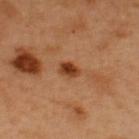- tile lighting: cross-polarized
- patient: male, about 50 years old
- automated metrics: a mean CIELAB color near L≈41 a*≈26 b*≈36, about 13 CIELAB-L* units darker than the surrounding skin, and a normalized border contrast of about 10.5; a within-lesion color-variation index near 4/10 and radial color variation of about 1; a classifier nevus-likeness of about 95/100
- image source: ~15 mm crop, total-body skin-cancer survey
- site: the upper back